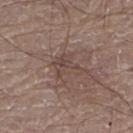{"biopsy_status": "not biopsied; imaged during a skin examination", "automated_metrics": {"area_mm2_approx": 6.5, "eccentricity": 0.75, "shape_asymmetry": 0.5, "border_irregularity_0_10": 6.0, "color_variation_0_10": 3.0, "peripheral_color_asymmetry": 1.0}, "image": {"source": "total-body photography crop", "field_of_view_mm": 15}, "lighting": "white-light", "site": "right leg", "patient": {"sex": "male", "age_approx": 80}, "lesion_size": {"long_diameter_mm_approx": 4.0}}